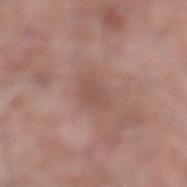workup — no biopsy performed (imaged during a skin exam) | subject — male, aged around 75 | automated metrics — border irregularity of about 2.5 on a 0–10 scale, a color-variation rating of about 1.5/10, and a peripheral color-asymmetry measure near 0.5; an automated nevus-likeness rating near 0 out of 100 and a detector confidence of about 100 out of 100 that the crop contains a lesion | site — the left lower leg | imaging modality — ~15 mm tile from a whole-body skin photo | illumination — white-light illumination.